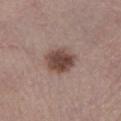biopsy_status: not biopsied; imaged during a skin examination
lesion_size:
  long_diameter_mm_approx: 4.0
automated_metrics:
  area_mm2_approx: 11.0
  eccentricity: 0.6
  border_irregularity_0_10: 1.5
  color_variation_0_10: 4.5
site: left lower leg
image:
  source: total-body photography crop
  field_of_view_mm: 15
lighting: white-light
patient:
  sex: male
  age_approx: 55